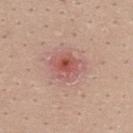| feature | finding |
|---|---|
| notes | imaged on a skin check; not biopsied |
| image | ~15 mm tile from a whole-body skin photo |
| illumination | white-light |
| site | the back |
| patient | female, aged 23 to 27 |
| size | about 4.5 mm |